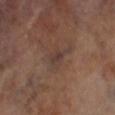Captured during whole-body skin photography for melanoma surveillance; the lesion was not biopsied.
The lesion is located on the leg.
Longest diameter approximately 3 mm.
A 15 mm crop from a total-body photograph taken for skin-cancer surveillance.
Captured under cross-polarized illumination.
The total-body-photography lesion software estimated a mean CIELAB color near L≈36 a*≈15 b*≈20 and a lesion-to-skin contrast of about 6.5 (normalized; higher = more distinct).
The subject is a male in their 70s.